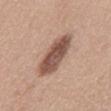follow-up: imaged on a skin check; not biopsied | image-analysis metrics: an eccentricity of roughly 0.9 and two-axis asymmetry of about 0.15; a lesion–skin lightness drop of about 15 and a normalized lesion–skin contrast near 10.5; a border-irregularity index near 2/10, internal color variation of about 4 on a 0–10 scale, and radial color variation of about 1.5; a nevus-likeness score of about 55/100 | location: the back | image source: 15 mm crop, total-body photography | diameter: about 6 mm | subject: male, aged 63 to 67 | illumination: white-light.